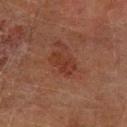biopsy_status: not biopsied; imaged during a skin examination
automated_metrics:
  area_mm2_approx: 5.5
  eccentricity: 0.85
  shape_asymmetry: 0.25
  cielab_L: 26
  cielab_a: 20
  cielab_b: 23
  vs_skin_contrast_norm: 6.0
  border_irregularity_0_10: 3.0
  color_variation_0_10: 2.5
  peripheral_color_asymmetry: 1.0
lighting: cross-polarized
site: left forearm
patient:
  sex: male
  age_approx: 60
image:
  source: total-body photography crop
  field_of_view_mm: 15
lesion_size:
  long_diameter_mm_approx: 3.5